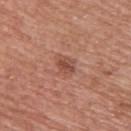| key | value |
|---|---|
| automated lesion analysis | a lesion area of about 5 mm², an outline eccentricity of about 0.65 (0 = round, 1 = elongated), and two-axis asymmetry of about 0.2; a border-irregularity index near 2/10 and internal color variation of about 3.5 on a 0–10 scale |
| patient | female, roughly 60 years of age |
| imaging modality | ~15 mm crop, total-body skin-cancer survey |
| location | the upper back |